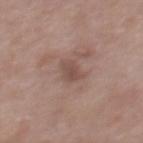The lesion was tiled from a total-body skin photograph and was not biopsied. On the upper back. The patient is a female aged approximately 50. A close-up tile cropped from a whole-body skin photograph, about 15 mm across.